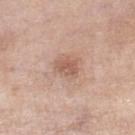This lesion was catalogued during total-body skin photography and was not selected for biopsy. Longest diameter approximately 3 mm. A female patient, about 55 years old. A 15 mm crop from a total-body photograph taken for skin-cancer surveillance. The tile uses white-light illumination. On the right lower leg.